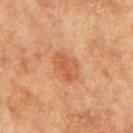A female patient, aged approximately 65. About 4 mm across. From the left arm. A 15 mm close-up tile from a total-body photography series done for melanoma screening. This is a cross-polarized tile.